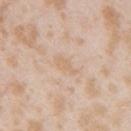biopsy_status: not biopsied; imaged during a skin examination
site: right upper arm
lesion_size:
  long_diameter_mm_approx: 3.0
lighting: white-light
image:
  source: total-body photography crop
  field_of_view_mm: 15
patient:
  sex: female
  age_approx: 25